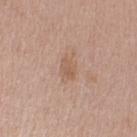No biopsy was performed on this lesion — it was imaged during a full skin examination and was not determined to be concerning. Longest diameter approximately 2.5 mm. On the arm. Automated image analysis of the tile measured an average lesion color of about L≈57 a*≈18 b*≈30 (CIELAB) and roughly 7 lightness units darker than nearby skin. The subject is a female aged approximately 40. This image is a 15 mm lesion crop taken from a total-body photograph. Imaged with white-light lighting.